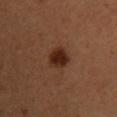Q: Where on the body is the lesion?
A: the chest
Q: What are the patient's age and sex?
A: male, aged around 35
Q: Lesion size?
A: ≈3.5 mm
Q: Automated lesion metrics?
A: a shape eccentricity near 0.65 and a shape-asymmetry score of about 0.2 (0 = symmetric); a border-irregularity rating of about 2/10, internal color variation of about 2.5 on a 0–10 scale, and radial color variation of about 0.5
Q: What kind of image is this?
A: total-body-photography crop, ~15 mm field of view
Q: Illumination type?
A: cross-polarized illumination